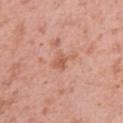{"biopsy_status": "not biopsied; imaged during a skin examination", "automated_metrics": {"area_mm2_approx": 3.5, "eccentricity": 0.75, "shape_asymmetry": 0.35, "vs_skin_darker_L": 8.0, "vs_skin_contrast_norm": 6.0, "nevus_likeness_0_100": 0, "lesion_detection_confidence_0_100": 100}, "lighting": "white-light", "site": "right upper arm", "lesion_size": {"long_diameter_mm_approx": 3.0}, "image": {"source": "total-body photography crop", "field_of_view_mm": 15}, "patient": {"sex": "female", "age_approx": 40}}